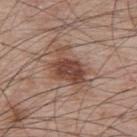  biopsy_status: not biopsied; imaged during a skin examination
  image:
    source: total-body photography crop
    field_of_view_mm: 15
  lighting: white-light
  patient:
    sex: male
    age_approx: 65
  site: upper back